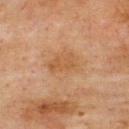Imaged during a routine full-body skin examination; the lesion was not biopsied and no histopathology is available.
A 15 mm close-up extracted from a 3D total-body photography capture.
The subject is a male in their mid-70s.
Located on the upper back.